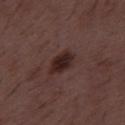Clinical impression: No biopsy was performed on this lesion — it was imaged during a full skin examination and was not determined to be concerning. Context: A male subject, in their 50s. About 3.5 mm across. A roughly 15 mm field-of-view crop from a total-body skin photograph. This is a white-light tile. Automated tile analysis of the lesion measured a border-irregularity index near 2/10, a color-variation rating of about 4/10, and a peripheral color-asymmetry measure near 1. The analysis additionally found a classifier nevus-likeness of about 85/100.